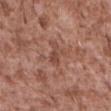Recorded during total-body skin imaging; not selected for excision or biopsy. On the abdomen. The subject is a male in their mid-40s. The lesion's longest dimension is about 3 mm. Automated tile analysis of the lesion measured a footprint of about 3 mm², an outline eccentricity of about 0.9 (0 = round, 1 = elongated), and a shape-asymmetry score of about 0.5 (0 = symmetric). The analysis additionally found a lesion color around L≈46 a*≈22 b*≈27 in CIELAB, about 8 CIELAB-L* units darker than the surrounding skin, and a lesion-to-skin contrast of about 6 (normalized; higher = more distinct). And it measured a border-irregularity rating of about 5/10, a within-lesion color-variation index near 0.5/10, and peripheral color asymmetry of about 0. The tile uses white-light illumination. A close-up tile cropped from a whole-body skin photograph, about 15 mm across.